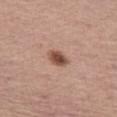  biopsy_status: not biopsied; imaged during a skin examination
  image:
    source: total-body photography crop
    field_of_view_mm: 15
  site: left thigh
  patient:
    sex: female
    age_approx: 65